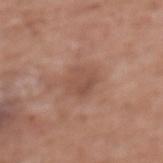A lesion tile, about 15 mm wide, cut from a 3D total-body photograph. A female subject in their mid-70s. Imaged with white-light lighting. Automated tile analysis of the lesion measured an area of roughly 7 mm² and an outline eccentricity of about 0.35 (0 = round, 1 = elongated). The analysis additionally found an average lesion color of about L≈50 a*≈21 b*≈27 (CIELAB) and a lesion–skin lightness drop of about 7. The software also gave peripheral color asymmetry of about 1. The software also gave an automated nevus-likeness rating near 0 out of 100 and a lesion-detection confidence of about 100/100. From the upper back. Approximately 3 mm at its widest.